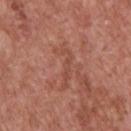Assessment:
Imaged during a routine full-body skin examination; the lesion was not biopsied and no histopathology is available.
Clinical summary:
The tile uses white-light illumination. A male subject, about 70 years old. A roughly 15 mm field-of-view crop from a total-body skin photograph. From the upper back. Approximately 5 mm at its widest.